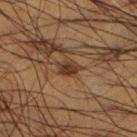Recorded during total-body skin imaging; not selected for excision or biopsy.
From the left lower leg.
A lesion tile, about 15 mm wide, cut from a 3D total-body photograph.
The total-body-photography lesion software estimated an average lesion color of about L≈27 a*≈15 b*≈25 (CIELAB), a lesion–skin lightness drop of about 8, and a normalized lesion–skin contrast near 9. It also reported an automated nevus-likeness rating near 90 out of 100 and a lesion-detection confidence of about 100/100.
This is a cross-polarized tile.
The patient is a male aged around 50.
The lesion's longest dimension is about 2.5 mm.